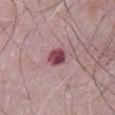Notes:
- follow-up — imaged on a skin check; not biopsied
- subject — male, roughly 65 years of age
- image — ~15 mm tile from a whole-body skin photo
- lesion size — ~3 mm (longest diameter)
- lighting — white-light
- site — the abdomen
- automated metrics — lesion-presence confidence of about 100/100